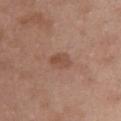| field | value |
|---|---|
| biopsy status | catalogued during a skin exam; not biopsied |
| illumination | white-light |
| image | ~15 mm tile from a whole-body skin photo |
| anatomic site | the chest |
| subject | female, about 50 years old |
| lesion size | ≈2.5 mm |
| automated lesion analysis | a lesion color around L≈49 a*≈20 b*≈28 in CIELAB; a border-irregularity rating of about 2/10, a within-lesion color-variation index near 2.5/10, and a peripheral color-asymmetry measure near 1; an automated nevus-likeness rating near 15 out of 100 and a detector confidence of about 100 out of 100 that the crop contains a lesion |